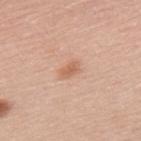{
  "biopsy_status": "not biopsied; imaged during a skin examination",
  "image": {
    "source": "total-body photography crop",
    "field_of_view_mm": 15
  },
  "lesion_size": {
    "long_diameter_mm_approx": 2.5
  },
  "patient": {
    "sex": "female",
    "age_approx": 65
  },
  "lighting": "white-light",
  "site": "back"
}